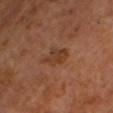Findings:
- notes — imaged on a skin check; not biopsied
- body site — the head or neck
- patient — male, roughly 50 years of age
- automated metrics — an average lesion color of about L≈37 a*≈22 b*≈31 (CIELAB), a lesion–skin lightness drop of about 7, and a lesion-to-skin contrast of about 6.5 (normalized; higher = more distinct); an automated nevus-likeness rating near 10 out of 100 and a detector confidence of about 100 out of 100 that the crop contains a lesion
- imaging modality — ~15 mm crop, total-body skin-cancer survey